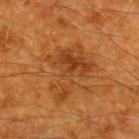Recorded during total-body skin imaging; not selected for excision or biopsy.
This is a cross-polarized tile.
A close-up tile cropped from a whole-body skin photograph, about 15 mm across.
The lesion is located on the back.
Automated image analysis of the tile measured an average lesion color of about L≈40 a*≈25 b*≈39 (CIELAB), roughly 8 lightness units darker than nearby skin, and a lesion-to-skin contrast of about 6.5 (normalized; higher = more distinct). And it measured internal color variation of about 6 on a 0–10 scale and a peripheral color-asymmetry measure near 2. And it measured a lesion-detection confidence of about 100/100.
About 6.5 mm across.
The patient is a male about 60 years old.